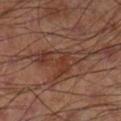workup — no biopsy performed (imaged during a skin exam) | lighting — cross-polarized illumination | lesion size — ~6.5 mm (longest diameter) | imaging modality — 15 mm crop, total-body photography | location — the right lower leg | automated lesion analysis — an average lesion color of about L≈38 a*≈21 b*≈27 (CIELAB), roughly 7 lightness units darker than nearby skin, and a lesion-to-skin contrast of about 6.5 (normalized; higher = more distinct); a color-variation rating of about 6/10 and peripheral color asymmetry of about 2; a lesion-detection confidence of about 90/100.